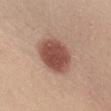notes: catalogued during a skin exam; not biopsied
lesion diameter: ≈5 mm
image: ~15 mm tile from a whole-body skin photo
location: the chest
subject: female, aged approximately 30
lighting: white-light illumination